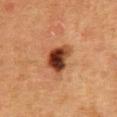Part of a total-body skin-imaging series; this lesion was reviewed on a skin check and was not flagged for biopsy. Measured at roughly 3.5 mm in maximum diameter. A 15 mm close-up extracted from a 3D total-body photography capture. The subject is a female in their 50s. On the abdomen.